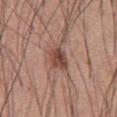follow-up: total-body-photography surveillance lesion; no biopsy
tile lighting: white-light
subject: male, aged around 60
lesion size: about 3.5 mm
body site: the abdomen
image-analysis metrics: a lesion area of about 7 mm², an eccentricity of roughly 0.6, and a symmetry-axis asymmetry near 0.3; border irregularity of about 2.5 on a 0–10 scale
imaging modality: total-body-photography crop, ~15 mm field of view